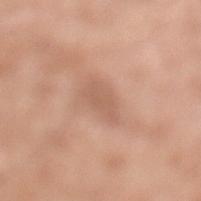Clinical impression: Imaged during a routine full-body skin examination; the lesion was not biopsied and no histopathology is available. Image and clinical context: The lesion-visualizer software estimated a lesion area of about 5.5 mm² and a symmetry-axis asymmetry near 0.35. The lesion's longest dimension is about 4 mm. A close-up tile cropped from a whole-body skin photograph, about 15 mm across. The lesion is on the leg. A male patient about 80 years old.